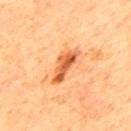  biopsy_status: not biopsied; imaged during a skin examination
  image:
    source: total-body photography crop
    field_of_view_mm: 15
  patient:
    sex: male
    age_approx: 65
  lesion_size:
    long_diameter_mm_approx: 5.0
  site: upper back